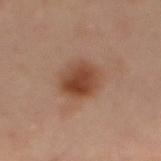biopsy_status: not biopsied; imaged during a skin examination
patient:
  sex: male
  age_approx: 50
lesion_size:
  long_diameter_mm_approx: 4.5
automated_metrics:
  shape_asymmetry: 0.15
  cielab_L: 44
  cielab_a: 21
  cielab_b: 30
  vs_skin_darker_L: 11.0
  vs_skin_contrast_norm: 9.0
  color_variation_0_10: 6.0
  nevus_likeness_0_100: 100
  lesion_detection_confidence_0_100: 100
image:
  source: total-body photography crop
  field_of_view_mm: 15
lighting: cross-polarized
site: mid back